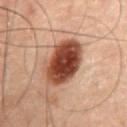Imaged during a routine full-body skin examination; the lesion was not biopsied and no histopathology is available. Cropped from a total-body skin-imaging series; the visible field is about 15 mm. From the abdomen. A male patient, in their 60s.